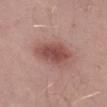Assessment:
Part of a total-body skin-imaging series; this lesion was reviewed on a skin check and was not flagged for biopsy.
Context:
The lesion is located on the left thigh. A male subject aged 48 to 52. A 15 mm crop from a total-body photograph taken for skin-cancer surveillance.